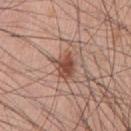Captured during whole-body skin photography for melanoma surveillance; the lesion was not biopsied.